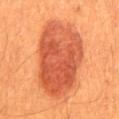biopsy status=catalogued during a skin exam; not biopsied | automated metrics=a nevus-likeness score of about 100/100 and a detector confidence of about 100 out of 100 that the crop contains a lesion | body site=the mid back | imaging modality=~15 mm crop, total-body skin-cancer survey | patient=male, aged approximately 60 | lighting=cross-polarized | size=≈10 mm.